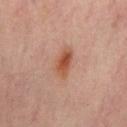notes = imaged on a skin check; not biopsied | subject = male, aged around 65 | anatomic site = the lower back | diameter = ~4 mm (longest diameter) | lighting = cross-polarized illumination | imaging modality = 15 mm crop, total-body photography | automated lesion analysis = a mean CIELAB color near L≈50 a*≈23 b*≈32, about 10 CIELAB-L* units darker than the surrounding skin, and a normalized border contrast of about 8.5; border irregularity of about 3.5 on a 0–10 scale, internal color variation of about 5.5 on a 0–10 scale, and radial color variation of about 2.